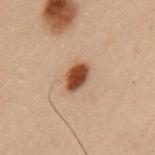Cropped from a whole-body photographic skin survey; the tile spans about 15 mm.
The total-body-photography lesion software estimated a shape eccentricity near 0.75. The analysis additionally found border irregularity of about 1.5 on a 0–10 scale and peripheral color asymmetry of about 1.5. The analysis additionally found a nevus-likeness score of about 100/100.
The lesion's longest dimension is about 3.5 mm.
A male subject, aged 53 to 57.
Located on the left upper arm.